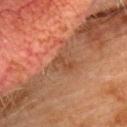Assessment:
Imaged during a routine full-body skin examination; the lesion was not biopsied and no histopathology is available.
Context:
The tile uses cross-polarized illumination. The lesion is on the chest. The total-body-photography lesion software estimated a footprint of about 4 mm², an eccentricity of roughly 0.8, and two-axis asymmetry of about 0.35. And it measured a lesion color around L≈39 a*≈20 b*≈29 in CIELAB, roughly 6 lightness units darker than nearby skin, and a lesion-to-skin contrast of about 5.5 (normalized; higher = more distinct). It also reported border irregularity of about 3.5 on a 0–10 scale, a within-lesion color-variation index near 2.5/10, and peripheral color asymmetry of about 1. It also reported a classifier nevus-likeness of about 0/100. A male subject, in their mid- to late 70s. A region of skin cropped from a whole-body photographic capture, roughly 15 mm wide.